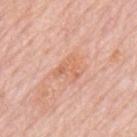Clinical impression:
Recorded during total-body skin imaging; not selected for excision or biopsy.
Image and clinical context:
A male patient, roughly 80 years of age. The tile uses white-light illumination. Automated image analysis of the tile measured an eccentricity of roughly 0.65 and a symmetry-axis asymmetry near 0.55. And it measured a border-irregularity index near 6.5/10 and radial color variation of about 1. The analysis additionally found an automated nevus-likeness rating near 0 out of 100 and lesion-presence confidence of about 100/100. Cropped from a whole-body photographic skin survey; the tile spans about 15 mm. From the mid back. Measured at roughly 3.5 mm in maximum diameter.